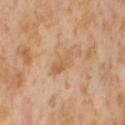The lesion was photographed on a routine skin check and not biopsied; there is no pathology result.
A 15 mm close-up tile from a total-body photography series done for melanoma screening.
The lesion is on the right thigh.
Measured at roughly 3.5 mm in maximum diameter.
The subject is a female in their mid-50s.